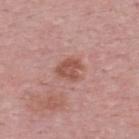Imaged during a routine full-body skin examination; the lesion was not biopsied and no histopathology is available. The recorded lesion diameter is about 3 mm. The lesion is on the upper back. A male subject aged approximately 50. The lesion-visualizer software estimated an average lesion color of about L≈52 a*≈25 b*≈27 (CIELAB), a lesion–skin lightness drop of about 10, and a normalized lesion–skin contrast near 7.5. And it measured internal color variation of about 2.5 on a 0–10 scale and a peripheral color-asymmetry measure near 1. Cropped from a total-body skin-imaging series; the visible field is about 15 mm. This is a white-light tile.